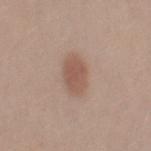Part of a total-body skin-imaging series; this lesion was reviewed on a skin check and was not flagged for biopsy.
This image is a 15 mm lesion crop taken from a total-body photograph.
The total-body-photography lesion software estimated a footprint of about 9 mm², a shape eccentricity near 0.8, and two-axis asymmetry of about 0.1. And it measured a mean CIELAB color near L≈54 a*≈19 b*≈26, about 9 CIELAB-L* units darker than the surrounding skin, and a lesion-to-skin contrast of about 6.5 (normalized; higher = more distinct). The analysis additionally found border irregularity of about 1.5 on a 0–10 scale. And it measured an automated nevus-likeness rating near 90 out of 100 and a lesion-detection confidence of about 100/100.
The patient is a female approximately 30 years of age.
On the right thigh.
Approximately 4.5 mm at its widest.